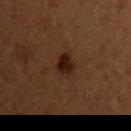Clinical impression:
Captured during whole-body skin photography for melanoma surveillance; the lesion was not biopsied.
Image and clinical context:
From the chest. A male subject in their 50s. A lesion tile, about 15 mm wide, cut from a 3D total-body photograph. About 3 mm across. Captured under cross-polarized illumination.